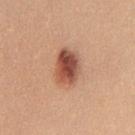Clinical impression:
The lesion was photographed on a routine skin check and not biopsied; there is no pathology result.
Context:
A female subject aged approximately 25. A roughly 15 mm field-of-view crop from a total-body skin photograph. The lesion is located on the chest. About 4 mm across.